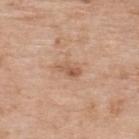  biopsy_status: not biopsied; imaged during a skin examination
  patient:
    sex: male
    age_approx: 70
  lesion_size:
    long_diameter_mm_approx: 3.0
  site: upper back
  lighting: white-light
  image:
    source: total-body photography crop
    field_of_view_mm: 15